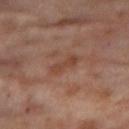Case summary:
– biopsy status — imaged on a skin check; not biopsied
– anatomic site — the right thigh
– automated lesion analysis — a shape eccentricity near 0.95 and a symmetry-axis asymmetry near 0.25
– image — ~15 mm crop, total-body skin-cancer survey
– subject — female, in their mid- to late 50s
– lesion diameter — about 3.5 mm